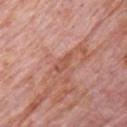Clinical summary:
A male patient roughly 80 years of age. Imaged with white-light lighting. The lesion is on the chest. This image is a 15 mm lesion crop taken from a total-body photograph. Measured at roughly 2.5 mm in maximum diameter. The total-body-photography lesion software estimated an area of roughly 3 mm², an outline eccentricity of about 0.9 (0 = round, 1 = elongated), and a symmetry-axis asymmetry near 0.3. The analysis additionally found internal color variation of about 1 on a 0–10 scale.